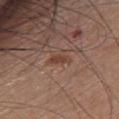Assessment: The lesion was photographed on a routine skin check and not biopsied; there is no pathology result. Background: Captured under white-light illumination. A female patient, about 65 years old. Longest diameter approximately 3 mm. The lesion is on the front of the torso. An algorithmic analysis of the crop reported a border-irregularity index near 3.5/10, internal color variation of about 0.5 on a 0–10 scale, and peripheral color asymmetry of about 0. It also reported an automated nevus-likeness rating near 5 out of 100. This image is a 15 mm lesion crop taken from a total-body photograph.